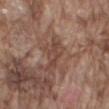workup=total-body-photography surveillance lesion; no biopsy | image=~15 mm crop, total-body skin-cancer survey | subject=male, roughly 75 years of age | tile lighting=white-light | anatomic site=the mid back.